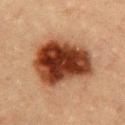Clinical impression: Captured during whole-body skin photography for melanoma surveillance; the lesion was not biopsied. Clinical summary: The lesion is on the chest. Approximately 7.5 mm at its widest. An algorithmic analysis of the crop reported a lesion color around L≈32 a*≈22 b*≈28 in CIELAB and a normalized border contrast of about 15.5. It also reported internal color variation of about 8 on a 0–10 scale. This image is a 15 mm lesion crop taken from a total-body photograph. Imaged with cross-polarized lighting. A female patient, approximately 55 years of age.